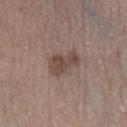Q: Is there a histopathology result?
A: catalogued during a skin exam; not biopsied
Q: What is the lesion's diameter?
A: ~4 mm (longest diameter)
Q: How was the tile lit?
A: white-light illumination
Q: How was this image acquired?
A: ~15 mm tile from a whole-body skin photo
Q: What are the patient's age and sex?
A: male, about 50 years old
Q: What is the anatomic site?
A: the leg